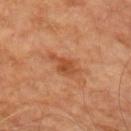Assessment: Captured during whole-body skin photography for melanoma surveillance; the lesion was not biopsied. Clinical summary: Captured under cross-polarized illumination. The lesion's longest dimension is about 4.5 mm. A close-up tile cropped from a whole-body skin photograph, about 15 mm across. On the right upper arm. The subject is a male in their 70s. The total-body-photography lesion software estimated border irregularity of about 5 on a 0–10 scale, a within-lesion color-variation index near 2.5/10, and radial color variation of about 0.5. The software also gave lesion-presence confidence of about 100/100.